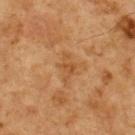Assessment:
Imaged during a routine full-body skin examination; the lesion was not biopsied and no histopathology is available.
Acquisition and patient details:
Automated tile analysis of the lesion measured a shape-asymmetry score of about 0.35 (0 = symmetric). And it measured a mean CIELAB color near L≈47 a*≈21 b*≈38. The analysis additionally found lesion-presence confidence of about 100/100. Measured at roughly 3 mm in maximum diameter. A male patient, aged approximately 60. A 15 mm close-up tile from a total-body photography series done for melanoma screening. Imaged with cross-polarized lighting. The lesion is located on the upper back.